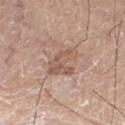A region of skin cropped from a whole-body photographic capture, roughly 15 mm wide. The lesion is located on the right thigh. The recorded lesion diameter is about 3.5 mm. Imaged with white-light lighting. A male patient, aged approximately 80. The total-body-photography lesion software estimated an area of roughly 9 mm², a shape eccentricity near 0.65, and a symmetry-axis asymmetry near 0.4. It also reported a border-irregularity rating of about 4.5/10.